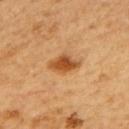{"biopsy_status": "not biopsied; imaged during a skin examination", "image": {"source": "total-body photography crop", "field_of_view_mm": 15}, "site": "back", "lesion_size": {"long_diameter_mm_approx": 3.5}, "lighting": "cross-polarized", "patient": {"sex": "male", "age_approx": 60}}